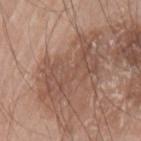Findings:
* workup — no biopsy performed (imaged during a skin exam)
* automated lesion analysis — an area of roughly 18 mm², an outline eccentricity of about 0.9 (0 = round, 1 = elongated), and two-axis asymmetry of about 0.45; a lesion color around L≈50 a*≈19 b*≈26 in CIELAB and a normalized border contrast of about 5.5; a color-variation rating of about 3/10 and peripheral color asymmetry of about 1
* patient — male, aged around 75
* lesion diameter — ≈7.5 mm
* site — the left upper arm
* illumination — white-light
* image source — ~15 mm tile from a whole-body skin photo